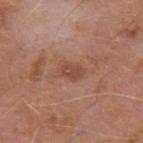notes: catalogued during a skin exam; not biopsied
image-analysis metrics: a lesion-to-skin contrast of about 6 (normalized; higher = more distinct); a border-irregularity rating of about 3/10; a classifier nevus-likeness of about 0/100 and lesion-presence confidence of about 100/100
acquisition: 15 mm crop, total-body photography
tile lighting: white-light
patient: male, about 65 years old
anatomic site: the left upper arm
size: about 3 mm